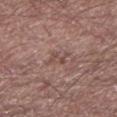Captured during whole-body skin photography for melanoma surveillance; the lesion was not biopsied. This is a white-light tile. Automated tile analysis of the lesion measured a lesion area of about 3.5 mm² and an eccentricity of roughly 0.8. And it measured border irregularity of about 5.5 on a 0–10 scale, internal color variation of about 0 on a 0–10 scale, and radial color variation of about 0. On the left lower leg. A male subject, about 55 years old. Measured at roughly 2.5 mm in maximum diameter. Cropped from a whole-body photographic skin survey; the tile spans about 15 mm.